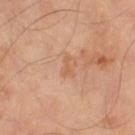{"biopsy_status": "not biopsied; imaged during a skin examination", "lighting": "cross-polarized", "patient": {"sex": "male", "age_approx": 65}, "site": "left thigh", "image": {"source": "total-body photography crop", "field_of_view_mm": 15}, "lesion_size": {"long_diameter_mm_approx": 2.5}}